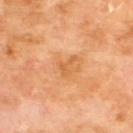The lesion is on the upper back.
Cropped from a total-body skin-imaging series; the visible field is about 15 mm.
Automated image analysis of the tile measured a lesion area of about 2.5 mm², an outline eccentricity of about 0.85 (0 = round, 1 = elongated), and a symmetry-axis asymmetry near 0.7. And it measured a border-irregularity index near 7/10, internal color variation of about 0 on a 0–10 scale, and radial color variation of about 0. The analysis additionally found a classifier nevus-likeness of about 0/100 and lesion-presence confidence of about 100/100.
Measured at roughly 3 mm in maximum diameter.
A male patient aged around 70.
This is a cross-polarized tile.